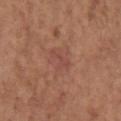Notes:
• body site — the left upper arm
• subject — female, aged approximately 65
• diameter — ~3 mm (longest diameter)
• illumination — white-light
• acquisition — ~15 mm crop, total-body skin-cancer survey